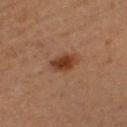Clinical impression: This lesion was catalogued during total-body skin photography and was not selected for biopsy. Image and clinical context: Imaged with cross-polarized lighting. The lesion is on the left upper arm. A 15 mm close-up extracted from a 3D total-body photography capture. Measured at roughly 3.5 mm in maximum diameter. A female patient about 60 years old.